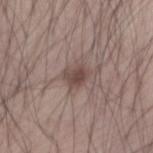| feature | finding |
|---|---|
| biopsy status | catalogued during a skin exam; not biopsied |
| illumination | white-light |
| image source | total-body-photography crop, ~15 mm field of view |
| TBP lesion metrics | a footprint of about 4.5 mm² and a shape eccentricity near 0.6; a lesion–skin lightness drop of about 10 and a normalized lesion–skin contrast near 8; an automated nevus-likeness rating near 65 out of 100 and lesion-presence confidence of about 100/100 |
| location | the left arm |
| patient | male, aged 68–72 |
| size | ≈2.5 mm |